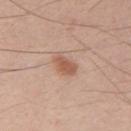Recorded during total-body skin imaging; not selected for excision or biopsy.
A region of skin cropped from a whole-body photographic capture, roughly 15 mm wide.
A male subject, approximately 35 years of age.
The lesion is on the right upper arm.